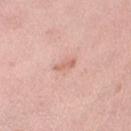Captured during whole-body skin photography for melanoma surveillance; the lesion was not biopsied.
A female subject, aged 63–67.
From the left lower leg.
Cropped from a total-body skin-imaging series; the visible field is about 15 mm.
Longest diameter approximately 2.5 mm.
Captured under white-light illumination.